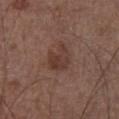Q: Was a biopsy performed?
A: catalogued during a skin exam; not biopsied
Q: Where on the body is the lesion?
A: the chest
Q: What lighting was used for the tile?
A: white-light illumination
Q: What did automated image analysis measure?
A: an eccentricity of roughly 0.55 and a symmetry-axis asymmetry near 0.35; a color-variation rating of about 3.5/10 and peripheral color asymmetry of about 1
Q: What are the patient's age and sex?
A: male, roughly 60 years of age
Q: How was this image acquired?
A: 15 mm crop, total-body photography